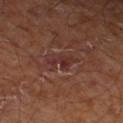Q: Was a biopsy performed?
A: total-body-photography surveillance lesion; no biopsy
Q: What lighting was used for the tile?
A: cross-polarized
Q: What kind of image is this?
A: total-body-photography crop, ~15 mm field of view
Q: Lesion location?
A: the left thigh
Q: What are the patient's age and sex?
A: about 65 years old
Q: Automated lesion metrics?
A: a lesion area of about 5.5 mm², a shape eccentricity near 0.8, and two-axis asymmetry of about 0.4; border irregularity of about 5.5 on a 0–10 scale, a within-lesion color-variation index near 3/10, and radial color variation of about 0.5; a classifier nevus-likeness of about 0/100